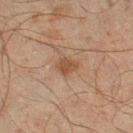Clinical impression:
This lesion was catalogued during total-body skin photography and was not selected for biopsy.
Context:
The total-body-photography lesion software estimated an outline eccentricity of about 0.6 (0 = round, 1 = elongated) and a symmetry-axis asymmetry near 0.25. And it measured a mean CIELAB color near L≈39 a*≈16 b*≈27, roughly 8 lightness units darker than nearby skin, and a lesion-to-skin contrast of about 7 (normalized; higher = more distinct). And it measured an automated nevus-likeness rating near 45 out of 100 and a lesion-detection confidence of about 100/100. A male patient, roughly 45 years of age. Captured under cross-polarized illumination. On the leg. A lesion tile, about 15 mm wide, cut from a 3D total-body photograph.